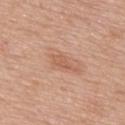Q: Was a biopsy performed?
A: total-body-photography surveillance lesion; no biopsy
Q: How large is the lesion?
A: ~3.5 mm (longest diameter)
Q: Lesion location?
A: the upper back
Q: What is the imaging modality?
A: total-body-photography crop, ~15 mm field of view
Q: What lighting was used for the tile?
A: white-light illumination
Q: Patient demographics?
A: male, roughly 75 years of age